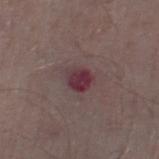Q: Was a biopsy performed?
A: no biopsy performed (imaged during a skin exam)
Q: Where on the body is the lesion?
A: the right thigh
Q: What is the imaging modality?
A: total-body-photography crop, ~15 mm field of view
Q: Patient demographics?
A: male, aged 53–57